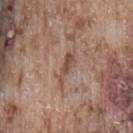image source — ~15 mm tile from a whole-body skin photo
anatomic site — the lower back
size — ≈4 mm
patient — male, in their mid-70s
lighting — white-light illumination